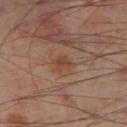Automated tile analysis of the lesion measured a lesion area of about 4 mm², an eccentricity of roughly 0.65, and a shape-asymmetry score of about 0.25 (0 = symmetric). It also reported a mean CIELAB color near L≈44 a*≈20 b*≈28, about 6 CIELAB-L* units darker than the surrounding skin, and a lesion-to-skin contrast of about 6 (normalized; higher = more distinct). And it measured a border-irregularity index near 2.5/10, a color-variation rating of about 2.5/10, and a peripheral color-asymmetry measure near 1.
Cropped from a whole-body photographic skin survey; the tile spans about 15 mm.
A male subject approximately 55 years of age.
This is a cross-polarized tile.
The lesion is located on the left thigh.
Approximately 2.5 mm at its widest.